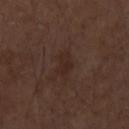Part of a total-body skin-imaging series; this lesion was reviewed on a skin check and was not flagged for biopsy.
About 3 mm across.
A male subject, aged around 75.
The lesion-visualizer software estimated an area of roughly 4.5 mm², an outline eccentricity of about 0.7 (0 = round, 1 = elongated), and two-axis asymmetry of about 0.35. It also reported a border-irregularity rating of about 3/10, a color-variation rating of about 1.5/10, and peripheral color asymmetry of about 0.5. And it measured a classifier nevus-likeness of about 0/100 and a lesion-detection confidence of about 100/100.
On the right forearm.
A lesion tile, about 15 mm wide, cut from a 3D total-body photograph.
The tile uses white-light illumination.